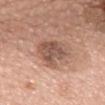The lesion was photographed on a routine skin check and not biopsied; there is no pathology result.
Imaged with white-light lighting.
A 15 mm close-up extracted from a 3D total-body photography capture.
An algorithmic analysis of the crop reported a lesion area of about 13 mm², an outline eccentricity of about 0.7 (0 = round, 1 = elongated), and a shape-asymmetry score of about 0.35 (0 = symmetric). And it measured a mean CIELAB color near L≈54 a*≈19 b*≈27, about 11 CIELAB-L* units darker than the surrounding skin, and a lesion-to-skin contrast of about 7.5 (normalized; higher = more distinct).
Located on the mid back.
The patient is a female aged 58–62.